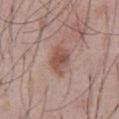This lesion was catalogued during total-body skin photography and was not selected for biopsy. The subject is a male aged approximately 55. Located on the abdomen. A region of skin cropped from a whole-body photographic capture, roughly 15 mm wide.